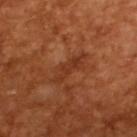| field | value |
|---|---|
| notes | catalogued during a skin exam; not biopsied |
| patient | male, roughly 65 years of age |
| acquisition | ~15 mm crop, total-body skin-cancer survey |
| tile lighting | cross-polarized illumination |